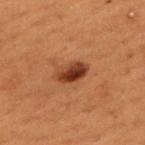biopsy status=total-body-photography surveillance lesion; no biopsy
subject=male, aged around 50
image source=15 mm crop, total-body photography
illumination=cross-polarized
anatomic site=the mid back